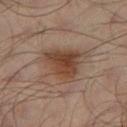workup = total-body-photography surveillance lesion; no biopsy | tile lighting = cross-polarized illumination | site = the left thigh | image = total-body-photography crop, ~15 mm field of view | patient = male, in their mid-60s | lesion size = about 5 mm.